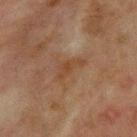Captured during whole-body skin photography for melanoma surveillance; the lesion was not biopsied. About 4 mm across. This image is a 15 mm lesion crop taken from a total-body photograph. The patient is a male aged around 65. The lesion is located on the chest.